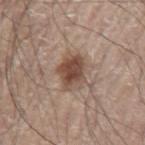notes — imaged on a skin check; not biopsied
lesion diameter — ≈4 mm
illumination — white-light
imaging modality — ~15 mm tile from a whole-body skin photo
subject — male, about 70 years old
anatomic site — the left upper arm